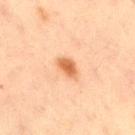Q: Illumination type?
A: cross-polarized illumination
Q: How was this image acquired?
A: total-body-photography crop, ~15 mm field of view
Q: Patient demographics?
A: male, aged around 70
Q: What is the lesion's diameter?
A: ~3 mm (longest diameter)
Q: What is the anatomic site?
A: the left thigh